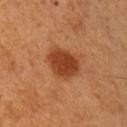Assessment:
The lesion was tiled from a total-body skin photograph and was not biopsied.
Acquisition and patient details:
Approximately 4 mm at its widest. A lesion tile, about 15 mm wide, cut from a 3D total-body photograph. Automated image analysis of the tile measured a lesion area of about 11 mm², an eccentricity of roughly 0.65, and a symmetry-axis asymmetry near 0.15. The software also gave a border-irregularity rating of about 1.5/10, a within-lesion color-variation index near 2.5/10, and peripheral color asymmetry of about 1. The software also gave a nevus-likeness score of about 100/100. The patient is a male aged around 50. Captured under cross-polarized illumination. From the arm.